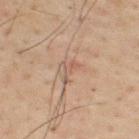Clinical summary: The recorded lesion diameter is about 2.5 mm. The lesion is on the upper back. The total-body-photography lesion software estimated a lesion color around L≈51 a*≈17 b*≈25 in CIELAB, about 7 CIELAB-L* units darker than the surrounding skin, and a normalized lesion–skin contrast near 5. The software also gave a nevus-likeness score of about 0/100 and a lesion-detection confidence of about 60/100. A lesion tile, about 15 mm wide, cut from a 3D total-body photograph. The patient is a male roughly 60 years of age.